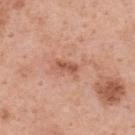Notes:
– notes — no biopsy performed (imaged during a skin exam)
– body site — the upper back
– illumination — white-light
– subject — female, aged 48 to 52
– size — about 2.5 mm
– imaging modality — 15 mm crop, total-body photography